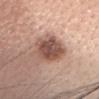{"biopsy_status": "not biopsied; imaged during a skin examination", "lighting": "white-light", "site": "head or neck", "image": {"source": "total-body photography crop", "field_of_view_mm": 15}, "lesion_size": {"long_diameter_mm_approx": 4.5}, "patient": {"sex": "female", "age_approx": 40}}